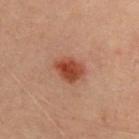Automated image analysis of the tile measured a mean CIELAB color near L≈36 a*≈24 b*≈27 and roughly 11 lightness units darker than nearby skin. The software also gave an automated nevus-likeness rating near 100 out of 100 and a detector confidence of about 100 out of 100 that the crop contains a lesion. Longest diameter approximately 3.5 mm. The tile uses cross-polarized illumination. The lesion is on the upper back. A female subject about 30 years old. A 15 mm close-up extracted from a 3D total-body photography capture.